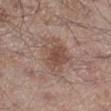The lesion is on the right lower leg. A region of skin cropped from a whole-body photographic capture, roughly 15 mm wide. The lesion's longest dimension is about 4.5 mm. Imaged with white-light lighting. A male subject, approximately 55 years of age.